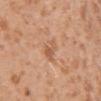{"biopsy_status": "not biopsied; imaged during a skin examination", "image": {"source": "total-body photography crop", "field_of_view_mm": 15}, "lighting": "white-light", "lesion_size": {"long_diameter_mm_approx": 2.5}, "patient": {"sex": "female", "age_approx": 30}, "site": "right upper arm"}